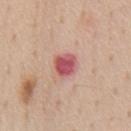follow-up: imaged on a skin check; not biopsied
automated lesion analysis: a footprint of about 6 mm², an outline eccentricity of about 0.3 (0 = round, 1 = elongated), and two-axis asymmetry of about 0.2
anatomic site: the chest
subject: male, approximately 55 years of age
imaging modality: total-body-photography crop, ~15 mm field of view